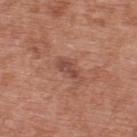Impression:
Recorded during total-body skin imaging; not selected for excision or biopsy.
Clinical summary:
The patient is a male in their 70s. The lesion is on the back. A close-up tile cropped from a whole-body skin photograph, about 15 mm across. This is a white-light tile. The recorded lesion diameter is about 3.5 mm.